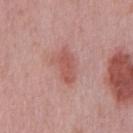| key | value |
|---|---|
| follow-up | catalogued during a skin exam; not biopsied |
| image | ~15 mm tile from a whole-body skin photo |
| size | ≈4 mm |
| lighting | white-light illumination |
| location | the chest |
| subject | male, roughly 50 years of age |
| TBP lesion metrics | a lesion–skin lightness drop of about 9 and a normalized lesion–skin contrast near 6.5; a border-irregularity rating of about 2.5/10, a within-lesion color-variation index near 2.5/10, and a peripheral color-asymmetry measure near 1; an automated nevus-likeness rating near 60 out of 100 and lesion-presence confidence of about 100/100 |